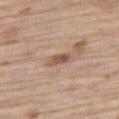The lesion was tiled from a total-body skin photograph and was not biopsied.
A male patient aged approximately 70.
The recorded lesion diameter is about 3 mm.
The tile uses white-light illumination.
An algorithmic analysis of the crop reported a lesion area of about 4 mm² and two-axis asymmetry of about 0.35. It also reported a lesion color around L≈54 a*≈18 b*≈28 in CIELAB, about 11 CIELAB-L* units darker than the surrounding skin, and a normalized border contrast of about 7.5. The analysis additionally found an automated nevus-likeness rating near 0 out of 100.
This image is a 15 mm lesion crop taken from a total-body photograph.
The lesion is located on the right thigh.